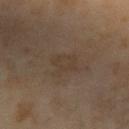Acquisition and patient details: The tile uses cross-polarized illumination. This image is a 15 mm lesion crop taken from a total-body photograph. The patient is a female in their 60s. Automated image analysis of the tile measured an average lesion color of about L≈33 a*≈11 b*≈23 (CIELAB) and a lesion-to-skin contrast of about 4.5 (normalized; higher = more distinct). The analysis additionally found a nevus-likeness score of about 0/100 and a detector confidence of about 100 out of 100 that the crop contains a lesion. The lesion is located on the left forearm.